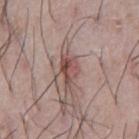Findings:
- workup · total-body-photography surveillance lesion; no biopsy
- acquisition · total-body-photography crop, ~15 mm field of view
- site · the abdomen
- patient · male, about 75 years old